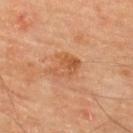Imaged during a routine full-body skin examination; the lesion was not biopsied and no histopathology is available. The lesion is located on the upper back. Cropped from a whole-body photographic skin survey; the tile spans about 15 mm. Approximately 3.5 mm at its widest. A male patient aged 63–67.